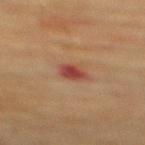Q: Was this lesion biopsied?
A: imaged on a skin check; not biopsied
Q: Lesion size?
A: ≈2.5 mm
Q: What lighting was used for the tile?
A: cross-polarized
Q: What kind of image is this?
A: ~15 mm tile from a whole-body skin photo
Q: What did automated image analysis measure?
A: an area of roughly 4.5 mm², an eccentricity of roughly 0.65, and a shape-asymmetry score of about 0.25 (0 = symmetric)
Q: What are the patient's age and sex?
A: female, aged 78 to 82
Q: What is the anatomic site?
A: the upper back